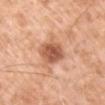No biopsy was performed on this lesion — it was imaged during a full skin examination and was not determined to be concerning.
From the left upper arm.
Cropped from a whole-body photographic skin survey; the tile spans about 15 mm.
The total-body-photography lesion software estimated a border-irregularity rating of about 2/10, a color-variation rating of about 4.5/10, and a peripheral color-asymmetry measure near 1.5. And it measured a detector confidence of about 100 out of 100 that the crop contains a lesion.
The lesion's longest dimension is about 3.5 mm.
A male patient, aged approximately 60.
Captured under white-light illumination.